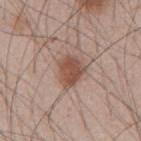Assessment: Part of a total-body skin-imaging series; this lesion was reviewed on a skin check and was not flagged for biopsy. Acquisition and patient details: Located on the abdomen. A roughly 15 mm field-of-view crop from a total-body skin photograph. About 4 mm across. The subject is a male roughly 45 years of age. Automated tile analysis of the lesion measured roughly 12 lightness units darker than nearby skin and a normalized border contrast of about 8.5. The analysis additionally found border irregularity of about 3 on a 0–10 scale and peripheral color asymmetry of about 1. The analysis additionally found an automated nevus-likeness rating near 95 out of 100 and lesion-presence confidence of about 100/100.